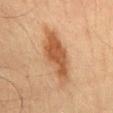A male subject in their mid-60s.
Cropped from a whole-body photographic skin survey; the tile spans about 15 mm.
From the abdomen.
An algorithmic analysis of the crop reported an average lesion color of about L≈45 a*≈20 b*≈32 (CIELAB), a lesion–skin lightness drop of about 11, and a normalized border contrast of about 8.5. The software also gave a border-irregularity rating of about 3.5/10, internal color variation of about 3.5 on a 0–10 scale, and peripheral color asymmetry of about 1. And it measured an automated nevus-likeness rating near 90 out of 100 and a detector confidence of about 100 out of 100 that the crop contains a lesion.